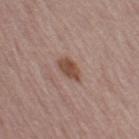notes: total-body-photography surveillance lesion; no biopsy | image: ~15 mm tile from a whole-body skin photo | location: the right thigh | automated lesion analysis: a footprint of about 5 mm²; a lesion color around L≈48 a*≈20 b*≈26 in CIELAB, a lesion–skin lightness drop of about 11, and a lesion-to-skin contrast of about 8.5 (normalized; higher = more distinct); a nevus-likeness score of about 70/100 and a detector confidence of about 100 out of 100 that the crop contains a lesion | subject: male, in their mid-60s.